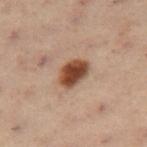Clinical impression:
This lesion was catalogued during total-body skin photography and was not selected for biopsy.
Clinical summary:
A 15 mm crop from a total-body photograph taken for skin-cancer surveillance. A female patient aged around 55. From the left leg.